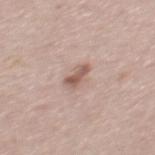| field | value |
|---|---|
| workup | no biopsy performed (imaged during a skin exam) |
| site | the mid back |
| image | 15 mm crop, total-body photography |
| patient | male, approximately 45 years of age |
| illumination | white-light |
| image-analysis metrics | a footprint of about 4.5 mm², an outline eccentricity of about 0.85 (0 = round, 1 = elongated), and a symmetry-axis asymmetry near 0.25; a nevus-likeness score of about 30/100 and lesion-presence confidence of about 100/100 |
| size | about 3 mm |